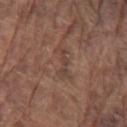biopsy status=total-body-photography surveillance lesion; no biopsy
lighting=white-light
automated metrics=an area of roughly 3.5 mm² and an eccentricity of roughly 0.95; a border-irregularity rating of about 8/10, a color-variation rating of about 0/10, and peripheral color asymmetry of about 0; a classifier nevus-likeness of about 0/100 and a lesion-detection confidence of about 55/100
diameter=about 3.5 mm
patient=male, roughly 80 years of age
imaging modality=15 mm crop, total-body photography
anatomic site=the arm